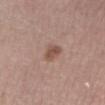| feature | finding |
|---|---|
| anatomic site | the leg |
| subject | male, aged 63 to 67 |
| image | 15 mm crop, total-body photography |